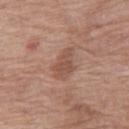No biopsy was performed on this lesion — it was imaged during a full skin examination and was not determined to be concerning. Approximately 4 mm at its widest. Located on the leg. A female patient, in their 70s. A lesion tile, about 15 mm wide, cut from a 3D total-body photograph. Captured under white-light illumination. Automated image analysis of the tile measured a footprint of about 7 mm², an outline eccentricity of about 0.85 (0 = round, 1 = elongated), and a symmetry-axis asymmetry near 0.25. And it measured an average lesion color of about L≈51 a*≈21 b*≈28 (CIELAB), about 8 CIELAB-L* units darker than the surrounding skin, and a normalized border contrast of about 6.5. The analysis additionally found a nevus-likeness score of about 0/100.